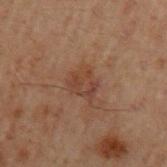Q: Was a biopsy performed?
A: catalogued during a skin exam; not biopsied
Q: Where on the body is the lesion?
A: the arm
Q: How was the tile lit?
A: cross-polarized
Q: What is the imaging modality?
A: ~15 mm crop, total-body skin-cancer survey
Q: How large is the lesion?
A: ≈3.5 mm
Q: Who is the patient?
A: male, aged 58–62